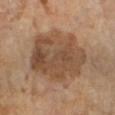Clinical impression: The lesion was tiled from a total-body skin photograph and was not biopsied. Clinical summary: Automated image analysis of the tile measured a mean CIELAB color near L≈45 a*≈17 b*≈29, about 10 CIELAB-L* units darker than the surrounding skin, and a normalized lesion–skin contrast near 8. The analysis additionally found an automated nevus-likeness rating near 45 out of 100. The recorded lesion diameter is about 8 mm. Cropped from a whole-body photographic skin survey; the tile spans about 15 mm. The lesion is located on the right lower leg. A female patient, in their 70s.